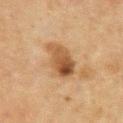The lesion was photographed on a routine skin check and not biopsied; there is no pathology result. Approximately 5.5 mm at its widest. A region of skin cropped from a whole-body photographic capture, roughly 15 mm wide. Imaged with cross-polarized lighting. Located on the front of the torso. A male patient aged around 65.